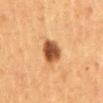Context:
The lesion is located on the abdomen. The recorded lesion diameter is about 3.5 mm. Cropped from a whole-body photographic skin survey; the tile spans about 15 mm. The total-body-photography lesion software estimated an area of roughly 7.5 mm² and a shape-asymmetry score of about 0.2 (0 = symmetric). And it measured a lesion color around L≈42 a*≈21 b*≈33 in CIELAB, roughly 16 lightness units darker than nearby skin, and a normalized lesion–skin contrast near 12.5. A female patient in their 50s. Imaged with cross-polarized lighting.